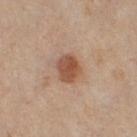{
  "site": "right thigh",
  "patient": {
    "sex": "female",
    "age_approx": 50
  },
  "automated_metrics": {
    "eccentricity": 0.65,
    "shape_asymmetry": 0.15,
    "cielab_L": 49,
    "cielab_a": 20,
    "cielab_b": 30,
    "vs_skin_contrast_norm": 8.5,
    "color_variation_0_10": 3.5,
    "peripheral_color_asymmetry": 1.0,
    "nevus_likeness_0_100": 100
  },
  "image": {
    "source": "total-body photography crop",
    "field_of_view_mm": 15
  }
}